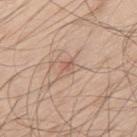{
  "biopsy_status": "not biopsied; imaged during a skin examination",
  "site": "upper back",
  "image": {
    "source": "total-body photography crop",
    "field_of_view_mm": 15
  },
  "patient": {
    "sex": "male",
    "age_approx": 70
  }
}